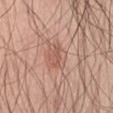| key | value |
|---|---|
| workup | no biopsy performed (imaged during a skin exam) |
| site | the abdomen |
| subject | male, approximately 55 years of age |
| acquisition | 15 mm crop, total-body photography |